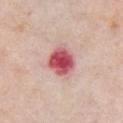The total-body-photography lesion software estimated a border-irregularity index near 1.5/10 and a within-lesion color-variation index near 7.5/10. It also reported an automated nevus-likeness rating near 0 out of 100 and a detector confidence of about 100 out of 100 that the crop contains a lesion. The subject is a female in their mid- to late 60s. The lesion is located on the chest. About 4 mm across. The tile uses white-light illumination. A region of skin cropped from a whole-body photographic capture, roughly 15 mm wide.